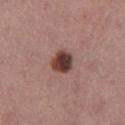No biopsy was performed on this lesion — it was imaged during a full skin examination and was not determined to be concerning.
Cropped from a whole-body photographic skin survey; the tile spans about 15 mm.
The subject is a female in their mid- to late 50s.
About 3 mm across.
The lesion is on the leg.